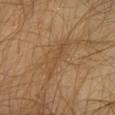workup = no biopsy performed (imaged during a skin exam) | diameter = ≈5.5 mm | lighting = cross-polarized illumination | body site = the chest | acquisition = ~15 mm tile from a whole-body skin photo | subject = male, about 30 years old.